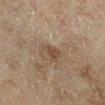Notes:
– notes · catalogued during a skin exam; not biopsied
– diameter · ≈3 mm
– lighting · cross-polarized
– image-analysis metrics · an area of roughly 3.5 mm² and two-axis asymmetry of about 0.35; a lesion color around L≈42 a*≈15 b*≈27 in CIELAB and a normalized lesion–skin contrast near 7; a border-irregularity rating of about 3.5/10 and internal color variation of about 1 on a 0–10 scale; a detector confidence of about 100 out of 100 that the crop contains a lesion
– site · the right lower leg
– subject · female, approximately 60 years of age
– acquisition · total-body-photography crop, ~15 mm field of view